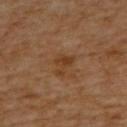An algorithmic analysis of the crop reported an area of roughly 4.5 mm² and a shape-asymmetry score of about 0.55 (0 = symmetric). And it measured a peripheral color-asymmetry measure near 0.5. A female patient approximately 65 years of age. Captured under cross-polarized illumination. The lesion is on the back. Cropped from a total-body skin-imaging series; the visible field is about 15 mm. The recorded lesion diameter is about 3 mm.